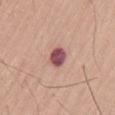Q: Is there a histopathology result?
A: catalogued during a skin exam; not biopsied
Q: What did automated image analysis measure?
A: a lesion area of about 5.5 mm² and an eccentricity of roughly 0.3; an average lesion color of about L≈51 a*≈26 b*≈19 (CIELAB) and a normalized border contrast of about 12; border irregularity of about 1.5 on a 0–10 scale, a within-lesion color-variation index near 3.5/10, and peripheral color asymmetry of about 1
Q: What kind of image is this?
A: 15 mm crop, total-body photography
Q: Who is the patient?
A: male, aged approximately 60
Q: Illumination type?
A: white-light
Q: What is the anatomic site?
A: the lower back
Q: How large is the lesion?
A: ~2.5 mm (longest diameter)